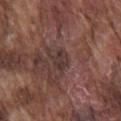Part of a total-body skin-imaging series; this lesion was reviewed on a skin check and was not flagged for biopsy.
A male patient roughly 75 years of age.
The lesion-visualizer software estimated an automated nevus-likeness rating near 0 out of 100 and lesion-presence confidence of about 95/100.
Longest diameter approximately 2.5 mm.
Captured under white-light illumination.
A roughly 15 mm field-of-view crop from a total-body skin photograph.
From the chest.